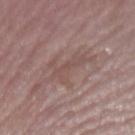biopsy status = total-body-photography surveillance lesion; no biopsy
tile lighting = white-light illumination
image = total-body-photography crop, ~15 mm field of view
image-analysis metrics = an area of roughly 7 mm² and two-axis asymmetry of about 0.6; a mean CIELAB color near L≈50 a*≈18 b*≈21, about 6 CIELAB-L* units darker than the surrounding skin, and a normalized lesion–skin contrast near 5; a color-variation rating of about 0.5/10 and radial color variation of about 0; a nevus-likeness score of about 0/100 and a detector confidence of about 50 out of 100 that the crop contains a lesion
patient = female, aged approximately 70
site = the left upper arm
diameter = ≈5.5 mm